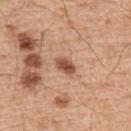Clinical impression: No biopsy was performed on this lesion — it was imaged during a full skin examination and was not determined to be concerning. Context: The tile uses white-light illumination. A male subject, aged 53–57. The lesion is located on the upper back. A close-up tile cropped from a whole-body skin photograph, about 15 mm across. The total-body-photography lesion software estimated an eccentricity of roughly 0.75 and a shape-asymmetry score of about 0.15 (0 = symmetric). It also reported a classifier nevus-likeness of about 95/100.